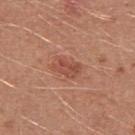  biopsy_status: not biopsied; imaged during a skin examination
  patient:
    sex: male
    age_approx: 25
  image:
    source: total-body photography crop
    field_of_view_mm: 15
  site: right upper arm